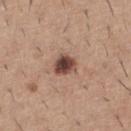Impression: No biopsy was performed on this lesion — it was imaged during a full skin examination and was not determined to be concerning.